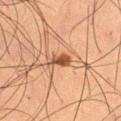Captured during whole-body skin photography for melanoma surveillance; the lesion was not biopsied. A male patient aged approximately 50. The lesion is on the right thigh. A lesion tile, about 15 mm wide, cut from a 3D total-body photograph. Imaged with cross-polarized lighting.